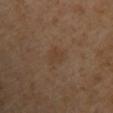Assessment: Part of a total-body skin-imaging series; this lesion was reviewed on a skin check and was not flagged for biopsy. Acquisition and patient details: A 15 mm close-up tile from a total-body photography series done for melanoma screening. Automated image analysis of the tile measured a border-irregularity index near 2.5/10, internal color variation of about 1 on a 0–10 scale, and radial color variation of about 0.5. It also reported a classifier nevus-likeness of about 0/100. The recorded lesion diameter is about 3 mm. A male patient, aged approximately 50. From the upper back.